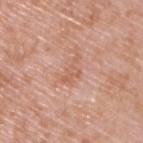| feature | finding |
|---|---|
| biopsy status | imaged on a skin check; not biopsied |
| lighting | white-light illumination |
| lesion diameter | ≈3.5 mm |
| automated lesion analysis | a mean CIELAB color near L≈60 a*≈23 b*≈31; internal color variation of about 1 on a 0–10 scale |
| site | the upper back |
| imaging modality | ~15 mm tile from a whole-body skin photo |
| patient | male, in their 50s |